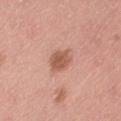Assessment:
Imaged during a routine full-body skin examination; the lesion was not biopsied and no histopathology is available.
Background:
Measured at roughly 3.5 mm in maximum diameter. A roughly 15 mm field-of-view crop from a total-body skin photograph. On the lower back. A male subject, aged approximately 40. Imaged with white-light lighting.